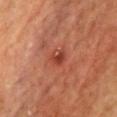Part of a total-body skin-imaging series; this lesion was reviewed on a skin check and was not flagged for biopsy.